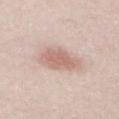| key | value |
|---|---|
| tile lighting | white-light |
| body site | the mid back |
| subject | male, in their mid-20s |
| diameter | about 4.5 mm |
| imaging modality | ~15 mm crop, total-body skin-cancer survey |